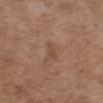Q: Lesion location?
A: the arm
Q: Who is the patient?
A: male, approximately 50 years of age
Q: What is the imaging modality?
A: 15 mm crop, total-body photography
Q: How large is the lesion?
A: about 2.5 mm
Q: What lighting was used for the tile?
A: white-light illumination